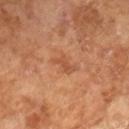{
  "biopsy_status": "not biopsied; imaged during a skin examination",
  "patient": {
    "sex": "male",
    "age_approx": 65
  },
  "image": {
    "source": "total-body photography crop",
    "field_of_view_mm": 15
  },
  "automated_metrics": {
    "area_mm2_approx": 3.5,
    "eccentricity": 0.85,
    "shape_asymmetry": 0.55,
    "cielab_L": 51,
    "cielab_a": 25,
    "cielab_b": 36,
    "vs_skin_darker_L": 7.0,
    "color_variation_0_10": 0.5,
    "nevus_likeness_0_100": 30
  },
  "lighting": "cross-polarized"
}